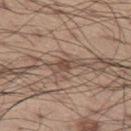Imaged during a routine full-body skin examination; the lesion was not biopsied and no histopathology is available. About 4 mm across. A male patient approximately 55 years of age. The lesion is on the left thigh. Cropped from a whole-body photographic skin survey; the tile spans about 15 mm.